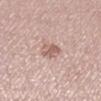Impression: This lesion was catalogued during total-body skin photography and was not selected for biopsy. Image and clinical context: This image is a 15 mm lesion crop taken from a total-body photograph. The lesion is on the left lower leg. The patient is a female roughly 35 years of age.